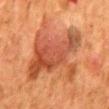No biopsy was performed on this lesion — it was imaged during a full skin examination and was not determined to be concerning. Captured under cross-polarized illumination. A close-up tile cropped from a whole-body skin photograph, about 15 mm across. From the mid back. A female subject, aged approximately 50. Longest diameter approximately 9 mm.